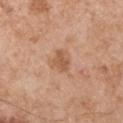Notes:
- biopsy status · total-body-photography surveillance lesion; no biopsy
- illumination · white-light
- subject · male, in their mid-50s
- size · ≈2.5 mm
- image-analysis metrics · a border-irregularity index near 2.5/10, a color-variation rating of about 1.5/10, and a peripheral color-asymmetry measure near 0.5
- imaging modality · ~15 mm crop, total-body skin-cancer survey
- site · the left upper arm